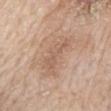Findings:
– notes — no biopsy performed (imaged during a skin exam)
– imaging modality — ~15 mm tile from a whole-body skin photo
– illumination — white-light
– image-analysis metrics — an area of roughly 6.5 mm², an outline eccentricity of about 0.9 (0 = round, 1 = elongated), and a symmetry-axis asymmetry near 0.55; roughly 7 lightness units darker than nearby skin and a normalized lesion–skin contrast near 5; a color-variation rating of about 2/10; a nevus-likeness score of about 0/100 and lesion-presence confidence of about 100/100
– anatomic site — the mid back
– patient — male, aged 63–67
– diameter — about 4.5 mm